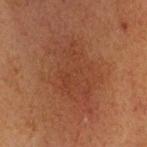Imaged during a routine full-body skin examination; the lesion was not biopsied and no histopathology is available. About 9.5 mm across. Automated tile analysis of the lesion measured an area of roughly 38 mm². The software also gave about 5 CIELAB-L* units darker than the surrounding skin and a normalized border contrast of about 5. The analysis additionally found border irregularity of about 5 on a 0–10 scale and a peripheral color-asymmetry measure near 1. It also reported a classifier nevus-likeness of about 0/100 and lesion-presence confidence of about 100/100. A male patient about 65 years old. Imaged with cross-polarized lighting. From the head or neck. A 15 mm close-up extracted from a 3D total-body photography capture.